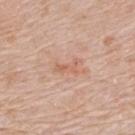No biopsy was performed on this lesion — it was imaged during a full skin examination and was not determined to be concerning. Imaged with white-light lighting. This image is a 15 mm lesion crop taken from a total-body photograph. A male patient aged approximately 65. On the back.